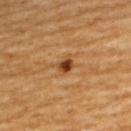Part of a total-body skin-imaging series; this lesion was reviewed on a skin check and was not flagged for biopsy. On the upper back. A male subject in their 60s. A 15 mm close-up tile from a total-body photography series done for melanoma screening.